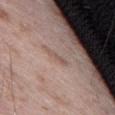Q: Was a biopsy performed?
A: total-body-photography surveillance lesion; no biopsy
Q: How was this image acquired?
A: total-body-photography crop, ~15 mm field of view
Q: Lesion location?
A: the right thigh
Q: Patient demographics?
A: male, aged approximately 70